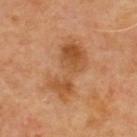notes: catalogued during a skin exam; not biopsied
location: the upper back
imaging modality: ~15 mm tile from a whole-body skin photo
subject: male, approximately 70 years of age